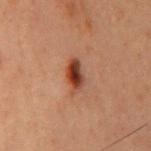Q: Where on the body is the lesion?
A: the mid back
Q: What is the imaging modality?
A: ~15 mm crop, total-body skin-cancer survey
Q: What are the patient's age and sex?
A: male, in their 60s